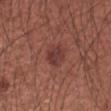biopsy_status: not biopsied; imaged during a skin examination
image:
  source: total-body photography crop
  field_of_view_mm: 15
site: right forearm
lesion_size:
  long_diameter_mm_approx: 3.0
lighting: white-light
patient:
  sex: male
  age_approx: 60